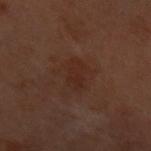Recorded during total-body skin imaging; not selected for excision or biopsy.
Approximately 3 mm at its widest.
Automated image analysis of the tile measured a classifier nevus-likeness of about 5/100.
From the front of the torso.
A female patient aged 58 to 62.
Cropped from a whole-body photographic skin survey; the tile spans about 15 mm.